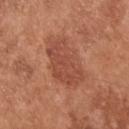Imaged during a routine full-body skin examination; the lesion was not biopsied and no histopathology is available. Captured under white-light illumination. A 15 mm close-up tile from a total-body photography series done for melanoma screening. On the upper back. A male subject in their mid-50s. Measured at roughly 6.5 mm in maximum diameter.